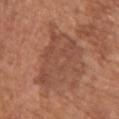workup: no biopsy performed (imaged during a skin exam) | body site: the chest | image source: total-body-photography crop, ~15 mm field of view | subject: male, aged approximately 65 | automated lesion analysis: roughly 7 lightness units darker than nearby skin and a normalized lesion–skin contrast near 5.5; a lesion-detection confidence of about 95/100 | tile lighting: white-light | diameter: ≈7.5 mm.